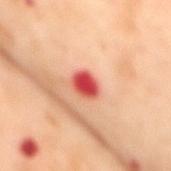{"image": {"source": "total-body photography crop", "field_of_view_mm": 15}, "automated_metrics": {"cielab_L": 57, "cielab_a": 45, "cielab_b": 35, "vs_skin_darker_L": 19.0, "vs_skin_contrast_norm": 11.5}, "patient": {"sex": "female", "age_approx": 55}, "lighting": "cross-polarized", "lesion_size": {"long_diameter_mm_approx": 3.0}, "site": "mid back"}